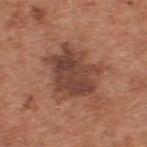Part of a total-body skin-imaging series; this lesion was reviewed on a skin check and was not flagged for biopsy.
The subject is a male aged 63–67.
The lesion is located on the upper back.
This is a white-light tile.
Automated image analysis of the tile measured a footprint of about 23 mm², an outline eccentricity of about 0.6 (0 = round, 1 = elongated), and a symmetry-axis asymmetry near 0.35.
A roughly 15 mm field-of-view crop from a total-body skin photograph.
Longest diameter approximately 7 mm.